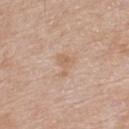Assessment:
This lesion was catalogued during total-body skin photography and was not selected for biopsy.
Background:
A male patient, aged around 80. Measured at roughly 3 mm in maximum diameter. A 15 mm crop from a total-body photograph taken for skin-cancer surveillance. Located on the upper back.